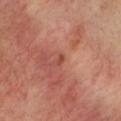notes — total-body-photography surveillance lesion; no biopsy
lesion diameter — ~1 mm (longest diameter)
location — the chest
patient — female, aged approximately 55
illumination — cross-polarized illumination
imaging modality — ~15 mm crop, total-body skin-cancer survey